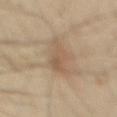biopsy_status: not biopsied; imaged during a skin examination
lesion_size:
  long_diameter_mm_approx: 4.5
site: mid back
lighting: cross-polarized
patient:
  sex: male
  age_approx: 55
image:
  source: total-body photography crop
  field_of_view_mm: 15